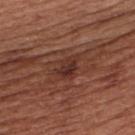Notes:
– biopsy status: imaged on a skin check; not biopsied
– subject: male, roughly 65 years of age
– anatomic site: the upper back
– lesion diameter: ~3.5 mm (longest diameter)
– illumination: white-light
– image source: ~15 mm crop, total-body skin-cancer survey